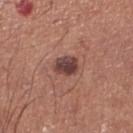Notes:
• notes · imaged on a skin check; not biopsied
• imaging modality · ~15 mm crop, total-body skin-cancer survey
• anatomic site · the right lower leg
• size · ≈3 mm
• lighting · white-light
• patient · male, approximately 70 years of age
• image-analysis metrics · a lesion area of about 5.5 mm², an eccentricity of roughly 0.65, and a shape-asymmetry score of about 0.2 (0 = symmetric); a border-irregularity rating of about 2/10, a within-lesion color-variation index near 4.5/10, and a peripheral color-asymmetry measure near 1.5